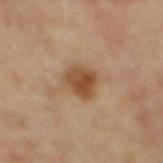| key | value |
|---|---|
| workup | total-body-photography surveillance lesion; no biopsy |
| image-analysis metrics | an eccentricity of roughly 0.2 and two-axis asymmetry of about 0.2; roughly 11 lightness units darker than nearby skin; a border-irregularity index near 2/10, internal color variation of about 4.5 on a 0–10 scale, and radial color variation of about 1.5; lesion-presence confidence of about 100/100 |
| patient | male, in their mid-80s or older |
| imaging modality | 15 mm crop, total-body photography |
| illumination | cross-polarized illumination |
| diameter | about 3 mm |
| anatomic site | the mid back |